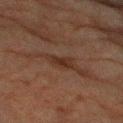Notes:
- notes — catalogued during a skin exam; not biopsied
- lighting — cross-polarized illumination
- anatomic site — the arm
- subject — male, aged approximately 80
- diameter — ~3.5 mm (longest diameter)
- imaging modality — 15 mm crop, total-body photography